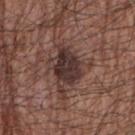follow-up: no biopsy performed (imaged during a skin exam) | acquisition: ~15 mm crop, total-body skin-cancer survey | body site: the right upper arm | illumination: white-light | patient: male, in their mid- to late 60s | diameter: ≈4.5 mm.